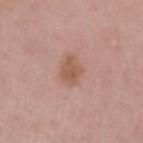Q: Was a biopsy performed?
A: total-body-photography surveillance lesion; no biopsy
Q: What lighting was used for the tile?
A: white-light illumination
Q: How was this image acquired?
A: ~15 mm tile from a whole-body skin photo
Q: Automated lesion metrics?
A: a border-irregularity index near 2.5/10, a color-variation rating of about 2/10, and peripheral color asymmetry of about 1; a nevus-likeness score of about 15/100 and lesion-presence confidence of about 100/100
Q: What is the lesion's diameter?
A: about 3.5 mm
Q: Who is the patient?
A: female, aged approximately 45
Q: What is the anatomic site?
A: the left upper arm